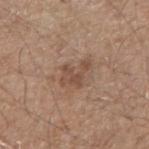  biopsy_status: not biopsied; imaged during a skin examination
  image:
    source: total-body photography crop
    field_of_view_mm: 15
  automated_metrics:
    eccentricity: 0.75
    shape_asymmetry: 0.5
    color_variation_0_10: 2.0
    nevus_likeness_0_100: 0
    lesion_detection_confidence_0_100: 100
  lighting: white-light
  site: right upper arm
  patient:
    sex: male
    age_approx: 65
  lesion_size:
    long_diameter_mm_approx: 3.5